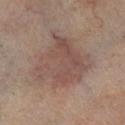Imaged during a routine full-body skin examination; the lesion was not biopsied and no histopathology is available. The patient is a male roughly 70 years of age. Located on the leg. This image is a 15 mm lesion crop taken from a total-body photograph.